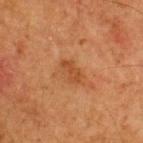{
  "biopsy_status": "not biopsied; imaged during a skin examination",
  "automated_metrics": {
    "eccentricity": 0.9,
    "vs_skin_darker_L": 7.0,
    "vs_skin_contrast_norm": 6.0,
    "border_irregularity_0_10": 3.0,
    "color_variation_0_10": 0.5,
    "peripheral_color_asymmetry": 0.0,
    "nevus_likeness_0_100": 0,
    "lesion_detection_confidence_0_100": 100
  },
  "patient": {
    "sex": "male",
    "age_approx": 65
  },
  "image": {
    "source": "total-body photography crop",
    "field_of_view_mm": 15
  },
  "site": "upper back",
  "lighting": "cross-polarized",
  "lesion_size": {
    "long_diameter_mm_approx": 3.0
  }
}